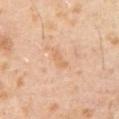The tile uses cross-polarized illumination.
A 15 mm crop from a total-body photograph taken for skin-cancer surveillance.
The total-body-photography lesion software estimated border irregularity of about 3.5 on a 0–10 scale, a within-lesion color-variation index near 0/10, and a peripheral color-asymmetry measure near 0.
The patient is a male about 65 years old.